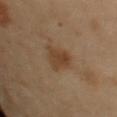Q: Was this lesion biopsied?
A: total-body-photography surveillance lesion; no biopsy
Q: What did automated image analysis measure?
A: an outline eccentricity of about 0.8 (0 = round, 1 = elongated) and a shape-asymmetry score of about 0.3 (0 = symmetric); an average lesion color of about L≈35 a*≈15 b*≈27 (CIELAB), about 7 CIELAB-L* units darker than the surrounding skin, and a lesion-to-skin contrast of about 7 (normalized; higher = more distinct); a classifier nevus-likeness of about 45/100 and a detector confidence of about 100 out of 100 that the crop contains a lesion
Q: How was this image acquired?
A: 15 mm crop, total-body photography
Q: Lesion location?
A: the right arm
Q: Illumination type?
A: cross-polarized illumination
Q: Who is the patient?
A: female, approximately 60 years of age